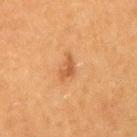No biopsy was performed on this lesion — it was imaged during a full skin examination and was not determined to be concerning.
A region of skin cropped from a whole-body photographic capture, roughly 15 mm wide.
Imaged with cross-polarized lighting.
The patient is a female in their 40s.
Located on the right upper arm.
The lesion's longest dimension is about 3.5 mm.
An algorithmic analysis of the crop reported a shape eccentricity near 0.9. The software also gave internal color variation of about 0 on a 0–10 scale and a peripheral color-asymmetry measure near 0.